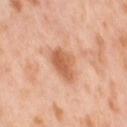Assessment:
The lesion was photographed on a routine skin check and not biopsied; there is no pathology result.
Context:
A female patient approximately 55 years of age. Cropped from a whole-body photographic skin survey; the tile spans about 15 mm. On the leg.